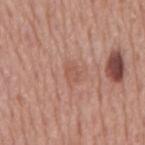Captured during whole-body skin photography for melanoma surveillance; the lesion was not biopsied. The lesion is on the mid back. A male subject aged 73 to 77. The recorded lesion diameter is about 2.5 mm. Captured under white-light illumination. Cropped from a whole-body photographic skin survey; the tile spans about 15 mm.